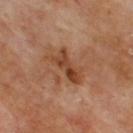| key | value |
|---|---|
| biopsy status | total-body-photography surveillance lesion; no biopsy |
| illumination | cross-polarized |
| subject | male, aged around 70 |
| lesion diameter | ~4.5 mm (longest diameter) |
| location | the back |
| image | total-body-photography crop, ~15 mm field of view |